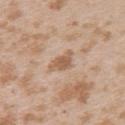- patient: female, in their mid-20s
- image source: 15 mm crop, total-body photography
- body site: the upper back